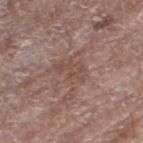follow-up — total-body-photography surveillance lesion; no biopsy | acquisition — ~15 mm tile from a whole-body skin photo | site — the leg | illumination — white-light illumination | image-analysis metrics — a nevus-likeness score of about 0/100 and a lesion-detection confidence of about 95/100 | patient — female, aged around 75 | lesion diameter — about 3.5 mm.